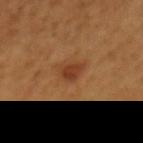Impression:
Imaged during a routine full-body skin examination; the lesion was not biopsied and no histopathology is available.
Context:
A roughly 15 mm field-of-view crop from a total-body skin photograph. A male patient, in their mid-40s. Located on the mid back.